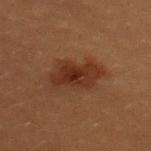No biopsy was performed on this lesion — it was imaged during a full skin examination and was not determined to be concerning. Captured under cross-polarized illumination. Cropped from a total-body skin-imaging series; the visible field is about 15 mm. The subject is a female about 20 years old. Located on the upper back. The total-body-photography lesion software estimated a footprint of about 13 mm² and an eccentricity of roughly 0.85. It also reported an average lesion color of about L≈24 a*≈18 b*≈24 (CIELAB), roughly 7 lightness units darker than nearby skin, and a lesion-to-skin contrast of about 8.5 (normalized; higher = more distinct). The analysis additionally found a classifier nevus-likeness of about 65/100 and a lesion-detection confidence of about 100/100.